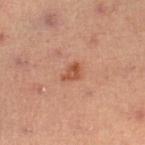{
  "biopsy_status": "not biopsied; imaged during a skin examination",
  "image": {
    "source": "total-body photography crop",
    "field_of_view_mm": 15
  },
  "patient": {
    "sex": "male",
    "age_approx": 55
  },
  "lighting": "cross-polarized",
  "site": "right lower leg"
}